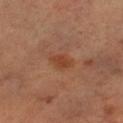image: ~15 mm tile from a whole-body skin photo | subject: female, about 60 years old | lesion size: ≈3 mm | location: the left lower leg | TBP lesion metrics: a lesion area of about 4.5 mm², an outline eccentricity of about 0.7 (0 = round, 1 = elongated), and a shape-asymmetry score of about 0.25 (0 = symmetric); a classifier nevus-likeness of about 30/100 and lesion-presence confidence of about 100/100 | illumination: cross-polarized.